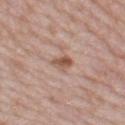  biopsy_status: not biopsied; imaged during a skin examination
  site: left thigh
  lesion_size:
    long_diameter_mm_approx: 2.5
  image:
    source: total-body photography crop
    field_of_view_mm: 15
  patient:
    sex: female
    age_approx: 60